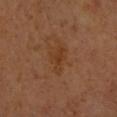<case>
<biopsy_status>not biopsied; imaged during a skin examination</biopsy_status>
<automated_metrics>
  <eccentricity>0.85</eccentricity>
  <shape_asymmetry>0.5</shape_asymmetry>
  <cielab_L>36</cielab_L>
  <cielab_a>22</cielab_a>
  <cielab_b>32</cielab_b>
  <vs_skin_darker_L>6.0</vs_skin_darker_L>
  <vs_skin_contrast_norm>6.0</vs_skin_contrast_norm>
  <border_irregularity_0_10>5.0</border_irregularity_0_10>
  <color_variation_0_10>1.0</color_variation_0_10>
  <peripheral_color_asymmetry>0.5</peripheral_color_asymmetry>
  <nevus_likeness_0_100>5</nevus_likeness_0_100>
  <lesion_detection_confidence_0_100>100</lesion_detection_confidence_0_100>
</automated_metrics>
<image>
  <source>total-body photography crop</source>
  <field_of_view_mm>15</field_of_view_mm>
</image>
<site>left forearm</site>
<patient>
  <sex>female</sex>
  <age_approx>40</age_approx>
</patient>
<lesion_size>
  <long_diameter_mm_approx>4.0</long_diameter_mm_approx>
</lesion_size>
</case>